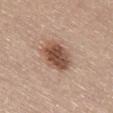Impression:
Captured during whole-body skin photography for melanoma surveillance; the lesion was not biopsied.
Clinical summary:
A 15 mm crop from a total-body photograph taken for skin-cancer surveillance. The lesion is located on the chest. A female subject aged around 65.